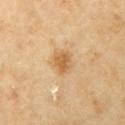Assessment:
Recorded during total-body skin imaging; not selected for excision or biopsy.
Clinical summary:
Automated tile analysis of the lesion measured a lesion color around L≈61 a*≈20 b*≈42 in CIELAB, about 10 CIELAB-L* units darker than the surrounding skin, and a lesion-to-skin contrast of about 7.5 (normalized; higher = more distinct). It also reported an automated nevus-likeness rating near 80 out of 100 and a detector confidence of about 100 out of 100 that the crop contains a lesion. Cropped from a whole-body photographic skin survey; the tile spans about 15 mm. A female subject, approximately 60 years of age. From the left upper arm.